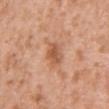No biopsy was performed on this lesion — it was imaged during a full skin examination and was not determined to be concerning. A roughly 15 mm field-of-view crop from a total-body skin photograph. A male patient, aged 28 to 32. Longest diameter approximately 3 mm. Imaged with white-light lighting. On the chest.